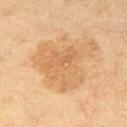{"biopsy_status": "not biopsied; imaged during a skin examination", "patient": {"sex": "female", "age_approx": 50}, "image": {"source": "total-body photography crop", "field_of_view_mm": 15}, "site": "right thigh", "lesion_size": {"long_diameter_mm_approx": 8.0}, "automated_metrics": {"area_mm2_approx": 30.0, "eccentricity": 0.55, "shape_asymmetry": 0.5, "border_irregularity_0_10": 6.0, "color_variation_0_10": 3.0, "peripheral_color_asymmetry": 1.0}}